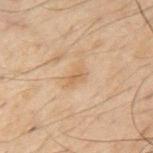Clinical impression: The lesion was tiled from a total-body skin photograph and was not biopsied. Background: A male patient, aged approximately 50. A 15 mm close-up tile from a total-body photography series done for melanoma screening. The lesion is located on the mid back.